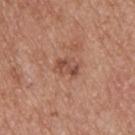Impression: Part of a total-body skin-imaging series; this lesion was reviewed on a skin check and was not flagged for biopsy. Context: Cropped from a whole-body photographic skin survey; the tile spans about 15 mm. The total-body-photography lesion software estimated a footprint of about 5 mm², an eccentricity of roughly 0.75, and a shape-asymmetry score of about 0.3 (0 = symmetric). It also reported a normalized border contrast of about 6.5. The analysis additionally found a classifier nevus-likeness of about 0/100 and a detector confidence of about 100 out of 100 that the crop contains a lesion. A male patient in their mid-50s. On the upper back. Imaged with white-light lighting. The lesion's longest dimension is about 3 mm.